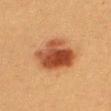No biopsy was performed on this lesion — it was imaged during a full skin examination and was not determined to be concerning.
Longest diameter approximately 5.5 mm.
A roughly 15 mm field-of-view crop from a total-body skin photograph.
Automated image analysis of the tile measured a lesion area of about 17 mm². It also reported a lesion color around L≈40 a*≈23 b*≈32 in CIELAB. And it measured a border-irregularity rating of about 2.5/10 and radial color variation of about 2.5. And it measured a nevus-likeness score of about 100/100.
A female patient, roughly 40 years of age.
Located on the right thigh.